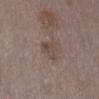Assessment:
Recorded during total-body skin imaging; not selected for excision or biopsy.
Acquisition and patient details:
Automated image analysis of the tile measured a footprint of about 5 mm², an outline eccentricity of about 0.8 (0 = round, 1 = elongated), and a shape-asymmetry score of about 0.45 (0 = symmetric). The analysis additionally found a mean CIELAB color near L≈46 a*≈14 b*≈21, about 7 CIELAB-L* units darker than the surrounding skin, and a normalized border contrast of about 6. And it measured a border-irregularity rating of about 4.5/10, a within-lesion color-variation index near 3/10, and radial color variation of about 1.5. The tile uses white-light illumination. Cropped from a total-body skin-imaging series; the visible field is about 15 mm. A female subject, roughly 35 years of age. The recorded lesion diameter is about 3 mm. Located on the abdomen.